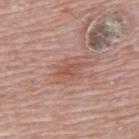follow-up = no biopsy performed (imaged during a skin exam) | lighting = white-light | TBP lesion metrics = a lesion–skin lightness drop of about 8; a color-variation rating of about 2/10 and peripheral color asymmetry of about 1; an automated nevus-likeness rating near 0 out of 100 and a detector confidence of about 100 out of 100 that the crop contains a lesion | image source = ~15 mm tile from a whole-body skin photo | patient = male, aged 68 to 72 | diameter = about 3 mm | anatomic site = the upper back.